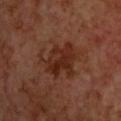Clinical impression: Imaged during a routine full-body skin examination; the lesion was not biopsied and no histopathology is available. Acquisition and patient details: The lesion is located on the upper back. The subject is a male aged 68–72. Imaged with cross-polarized lighting. A roughly 15 mm field-of-view crop from a total-body skin photograph. The lesion-visualizer software estimated an area of roughly 15 mm², a shape eccentricity near 0.7, and a shape-asymmetry score of about 0.35 (0 = symmetric). The analysis additionally found a lesion color around L≈27 a*≈22 b*≈27 in CIELAB, roughly 8 lightness units darker than nearby skin, and a lesion-to-skin contrast of about 8 (normalized; higher = more distinct). It also reported a color-variation rating of about 4.5/10 and radial color variation of about 1.5. The software also gave a classifier nevus-likeness of about 5/100 and lesion-presence confidence of about 100/100.